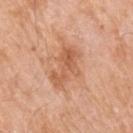Q: What is the anatomic site?
A: the arm
Q: How was the tile lit?
A: white-light illumination
Q: Who is the patient?
A: male, aged around 60
Q: What did automated image analysis measure?
A: a footprint of about 10 mm², an eccentricity of roughly 0.85, and a symmetry-axis asymmetry near 0.4; an average lesion color of about L≈59 a*≈25 b*≈36 (CIELAB) and a normalized lesion–skin contrast near 6.5; an automated nevus-likeness rating near 0 out of 100
Q: What is the imaging modality?
A: ~15 mm tile from a whole-body skin photo